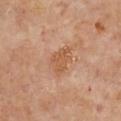No biopsy was performed on this lesion — it was imaged during a full skin examination and was not determined to be concerning. Longest diameter approximately 4 mm. A close-up tile cropped from a whole-body skin photograph, about 15 mm across. A female subject in their 60s. Located on the front of the torso. An algorithmic analysis of the crop reported an average lesion color of about L≈55 a*≈22 b*≈35 (CIELAB) and a lesion–skin lightness drop of about 8. The analysis additionally found an automated nevus-likeness rating near 5 out of 100 and lesion-presence confidence of about 100/100. Captured under cross-polarized illumination.